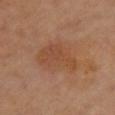<lesion>
  <biopsy_status>not biopsied; imaged during a skin examination</biopsy_status>
  <lesion_size>
    <long_diameter_mm_approx>6.0</long_diameter_mm_approx>
  </lesion_size>
  <patient>
    <sex>female</sex>
    <age_approx>40</age_approx>
  </patient>
  <site>chest</site>
  <image>
    <source>total-body photography crop</source>
    <field_of_view_mm>15</field_of_view_mm>
  </image>
  <lighting>cross-polarized</lighting>
</lesion>